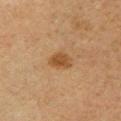notes=total-body-photography surveillance lesion; no biopsy | subject=female, in their 40s | illumination=cross-polarized | size=about 2.5 mm | image=~15 mm tile from a whole-body skin photo | anatomic site=the chest.